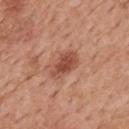patient:
  sex: male
  age_approx: 60
site: back
lighting: white-light
image:
  source: total-body photography crop
  field_of_view_mm: 15